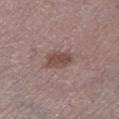follow-up — total-body-photography surveillance lesion; no biopsy
lighting — white-light
patient — male, aged approximately 70
anatomic site — the left lower leg
TBP lesion metrics — an area of roughly 6 mm², an outline eccentricity of about 0.75 (0 = round, 1 = elongated), and a symmetry-axis asymmetry near 0.2; a mean CIELAB color near L≈47 a*≈18 b*≈21, a lesion–skin lightness drop of about 10, and a normalized lesion–skin contrast near 8; internal color variation of about 2 on a 0–10 scale and radial color variation of about 1
acquisition — ~15 mm crop, total-body skin-cancer survey